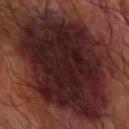notes: imaged on a skin check; not biopsied
anatomic site: the arm
subject: male, roughly 60 years of age
size: ~13.5 mm (longest diameter)
automated lesion analysis: border irregularity of about 3 on a 0–10 scale and a within-lesion color-variation index near 5.5/10; a nevus-likeness score of about 0/100 and a lesion-detection confidence of about 60/100
image source: 15 mm crop, total-body photography